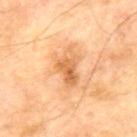Clinical impression: This lesion was catalogued during total-body skin photography and was not selected for biopsy. Background: Captured under cross-polarized illumination. From the mid back. The subject is a male aged approximately 70. Longest diameter approximately 4.5 mm. A lesion tile, about 15 mm wide, cut from a 3D total-body photograph.